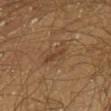Recorded during total-body skin imaging; not selected for excision or biopsy. A male patient, approximately 40 years of age. The lesion is on the chest. A 15 mm close-up tile from a total-body photography series done for melanoma screening. Approximately 2.5 mm at its widest. Automated image analysis of the tile measured roughly 7 lightness units darker than nearby skin and a normalized border contrast of about 6. The analysis additionally found a nevus-likeness score of about 0/100.